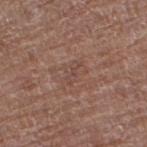Impression: Recorded during total-body skin imaging; not selected for excision or biopsy. Context: A female subject roughly 80 years of age. From the left thigh. A region of skin cropped from a whole-body photographic capture, roughly 15 mm wide.